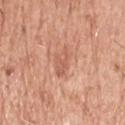  biopsy_status: not biopsied; imaged during a skin examination
  site: head or neck
  patient:
    sex: male
    age_approx: 70
  lesion_size:
    long_diameter_mm_approx: 4.0
  automated_metrics:
    vs_skin_darker_L: 8.0
    vs_skin_contrast_norm: 5.5
    border_irregularity_0_10: 3.5
    peripheral_color_asymmetry: 0.5
  image:
    source: total-body photography crop
    field_of_view_mm: 15